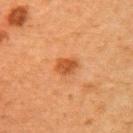Notes:
• automated metrics — an area of roughly 4.5 mm², an outline eccentricity of about 0.65 (0 = round, 1 = elongated), and two-axis asymmetry of about 0.2; a lesion color around L≈43 a*≈25 b*≈36 in CIELAB, about 10 CIELAB-L* units darker than the surrounding skin, and a normalized border contrast of about 8; a border-irregularity index near 2/10, a within-lesion color-variation index near 2.5/10, and radial color variation of about 1
• anatomic site — the right upper arm
• patient — female, aged approximately 60
• size — about 2.5 mm
• imaging modality — ~15 mm tile from a whole-body skin photo
• lighting — cross-polarized illumination